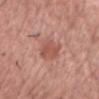biopsy status = catalogued during a skin exam; not biopsied
lighting = white-light
location = the front of the torso
subject = male, aged around 60
lesion diameter = ≈4 mm
image source = ~15 mm tile from a whole-body skin photo